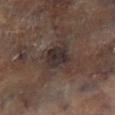biopsy_status: not biopsied; imaged during a skin examination
patient:
  sex: male
  age_approx: 65
site: leg
image:
  source: total-body photography crop
  field_of_view_mm: 15